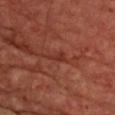workup: no biopsy performed (imaged during a skin exam) | patient: male, approximately 65 years of age | location: the chest | image: ~15 mm crop, total-body skin-cancer survey | lighting: cross-polarized.